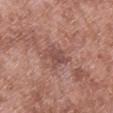Clinical summary: Imaged with white-light lighting. A male subject in their mid-50s. The recorded lesion diameter is about 3.5 mm. Located on the leg. Cropped from a total-body skin-imaging series; the visible field is about 15 mm. Automated tile analysis of the lesion measured a footprint of about 6 mm² and an eccentricity of roughly 0.7. It also reported a lesion color around L≈49 a*≈22 b*≈24 in CIELAB, a lesion–skin lightness drop of about 8, and a lesion-to-skin contrast of about 6 (normalized; higher = more distinct). The analysis additionally found a lesion-detection confidence of about 100/100.